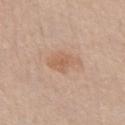follow-up = catalogued during a skin exam; not biopsied
image = ~15 mm crop, total-body skin-cancer survey
lesion size = ≈4 mm
patient = male, aged around 80
anatomic site = the chest
illumination = white-light illumination